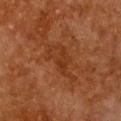No biopsy was performed on this lesion — it was imaged during a full skin examination and was not determined to be concerning.
A female patient aged approximately 50.
The lesion is on the chest.
This is a cross-polarized tile.
An algorithmic analysis of the crop reported a color-variation rating of about 2.5/10 and radial color variation of about 1. The software also gave a detector confidence of about 95 out of 100 that the crop contains a lesion.
A roughly 15 mm field-of-view crop from a total-body skin photograph.
The recorded lesion diameter is about 5.5 mm.